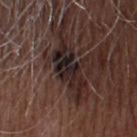| key | value |
|---|---|
| follow-up | imaged on a skin check; not biopsied |
| acquisition | total-body-photography crop, ~15 mm field of view |
| subject | male, in their 50s |
| anatomic site | the head or neck |
| lesion diameter | about 6.5 mm |
| tile lighting | white-light |